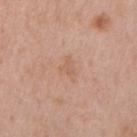Q: Was this lesion biopsied?
A: no biopsy performed (imaged during a skin exam)
Q: Automated lesion metrics?
A: an area of roughly 4 mm² and a shape-asymmetry score of about 0.55 (0 = symmetric); a mean CIELAB color near L≈60 a*≈20 b*≈31, about 5 CIELAB-L* units darker than the surrounding skin, and a normalized lesion–skin contrast near 4.5; an automated nevus-likeness rating near 0 out of 100 and a detector confidence of about 100 out of 100 that the crop contains a lesion
Q: Lesion location?
A: the back
Q: Who is the patient?
A: male, in their 70s
Q: How was the tile lit?
A: white-light illumination
Q: Lesion size?
A: ~3 mm (longest diameter)
Q: What kind of image is this?
A: total-body-photography crop, ~15 mm field of view